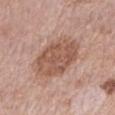{"biopsy_status": "not biopsied; imaged during a skin examination", "lighting": "white-light", "automated_metrics": {"color_variation_0_10": 3.5, "peripheral_color_asymmetry": 1.0}, "site": "right forearm", "patient": {"sex": "female", "age_approx": 65}, "image": {"source": "total-body photography crop", "field_of_view_mm": 15}}